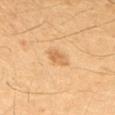Clinical impression: The lesion was photographed on a routine skin check and not biopsied; there is no pathology result. Image and clinical context: Imaged with cross-polarized lighting. The lesion's longest dimension is about 2.5 mm. A close-up tile cropped from a whole-body skin photograph, about 15 mm across. The lesion is located on the left upper arm. A male subject aged 43 to 47.